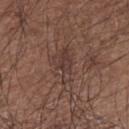Case summary:
– workup · no biopsy performed (imaged during a skin exam)
– subject · male, aged approximately 65
– lighting · white-light
– imaging modality · total-body-photography crop, ~15 mm field of view
– site · the left upper arm
– diameter · ≈4 mm